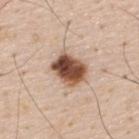Case summary:
- workup — imaged on a skin check; not biopsied
- size — ~4 mm (longest diameter)
- anatomic site — the upper back
- tile lighting — white-light illumination
- image source — 15 mm crop, total-body photography
- subject — male, aged 58–62